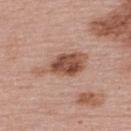Notes:
* biopsy status: catalogued during a skin exam; not biopsied
* anatomic site: the back
* subject: female, in their 50s
* image-analysis metrics: a mean CIELAB color near L≈51 a*≈22 b*≈28 and roughly 14 lightness units darker than nearby skin; a detector confidence of about 100 out of 100 that the crop contains a lesion
* lesion diameter: about 6 mm
* image: total-body-photography crop, ~15 mm field of view